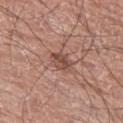Captured during whole-body skin photography for melanoma surveillance; the lesion was not biopsied. A 15 mm crop from a total-body photograph taken for skin-cancer surveillance. On the left thigh. The lesion's longest dimension is about 3 mm. Captured under white-light illumination. A male patient, aged 73–77.